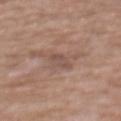patient:
  sex: female
  age_approx: 75
lesion_size:
  long_diameter_mm_approx: 3.0
site: mid back
image:
  source: total-body photography crop
  field_of_view_mm: 15
lighting: white-light